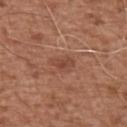Notes:
- notes: imaged on a skin check; not biopsied
- image: total-body-photography crop, ~15 mm field of view
- lesion size: ≈3 mm
- image-analysis metrics: a mean CIELAB color near L≈46 a*≈24 b*≈29, roughly 7 lightness units darker than nearby skin, and a lesion-to-skin contrast of about 5.5 (normalized; higher = more distinct); a classifier nevus-likeness of about 0/100 and lesion-presence confidence of about 100/100
- tile lighting: white-light
- anatomic site: the left upper arm
- patient: male, aged 73 to 77